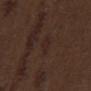Q: Is there a histopathology result?
A: imaged on a skin check; not biopsied
Q: What are the patient's age and sex?
A: male, in their 70s
Q: Where on the body is the lesion?
A: the mid back
Q: Automated lesion metrics?
A: a footprint of about 3.5 mm² and a shape eccentricity near 0.8; roughly 4 lightness units darker than nearby skin and a lesion-to-skin contrast of about 5 (normalized; higher = more distinct); a border-irregularity rating of about 2/10 and a within-lesion color-variation index near 2/10; a classifier nevus-likeness of about 0/100 and lesion-presence confidence of about 95/100
Q: How was the tile lit?
A: white-light illumination
Q: Lesion size?
A: about 3 mm
Q: How was this image acquired?
A: 15 mm crop, total-body photography